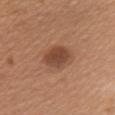The lesion was tiled from a total-body skin photograph and was not biopsied.